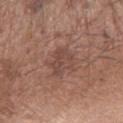Case summary:
• follow-up — no biopsy performed (imaged during a skin exam)
• location — the left forearm
• imaging modality — 15 mm crop, total-body photography
• lighting — white-light illumination
• patient — male, in their 70s
• lesion diameter — ~4 mm (longest diameter)
• image-analysis metrics — a lesion color around L≈46 a*≈20 b*≈24 in CIELAB, roughly 7 lightness units darker than nearby skin, and a normalized border contrast of about 5.5